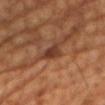Imaged during a routine full-body skin examination; the lesion was not biopsied and no histopathology is available. Approximately 3 mm at its widest. The lesion-visualizer software estimated an average lesion color of about L≈35 a*≈22 b*≈28 (CIELAB), roughly 10 lightness units darker than nearby skin, and a normalized border contrast of about 8.5. The analysis additionally found a border-irregularity index near 4/10, a color-variation rating of about 2.5/10, and peripheral color asymmetry of about 0.5. It also reported a nevus-likeness score of about 5/100 and a detector confidence of about 100 out of 100 that the crop contains a lesion. Imaged with cross-polarized lighting. A region of skin cropped from a whole-body photographic capture, roughly 15 mm wide. A male subject, in their mid-50s. On the chest.